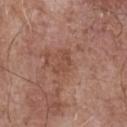Captured during whole-body skin photography for melanoma surveillance; the lesion was not biopsied. The lesion's longest dimension is about 3 mm. A male patient, approximately 70 years of age. A 15 mm close-up tile from a total-body photography series done for melanoma screening. Located on the chest. The tile uses white-light illumination.